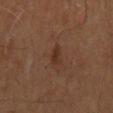Notes:
- notes · imaged on a skin check; not biopsied
- tile lighting · cross-polarized
- location · the front of the torso
- image source · total-body-photography crop, ~15 mm field of view
- patient · male, aged 58 to 62